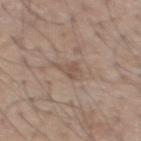biopsy status: imaged on a skin check; not biopsied | patient: male, roughly 55 years of age | lesion diameter: about 2.5 mm | lighting: white-light illumination | image source: ~15 mm tile from a whole-body skin photo | location: the upper back.